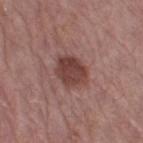Imaged during a routine full-body skin examination; the lesion was not biopsied and no histopathology is available. Captured under white-light illumination. Measured at roughly 4 mm in maximum diameter. A lesion tile, about 15 mm wide, cut from a 3D total-body photograph. On the right thigh. A female subject aged 63–67.